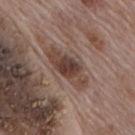Q: What lighting was used for the tile?
A: white-light illumination
Q: Who is the patient?
A: male, aged approximately 70
Q: Lesion location?
A: the mid back
Q: What kind of image is this?
A: ~15 mm tile from a whole-body skin photo